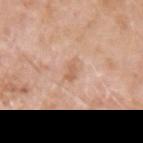The lesion was tiled from a total-body skin photograph and was not biopsied. A male patient aged 58 to 62. A 15 mm close-up tile from a total-body photography series done for melanoma screening. Approximately 2.5 mm at its widest. The lesion is on the left upper arm.